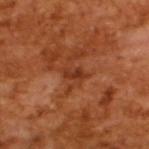Case summary:
• follow-up · catalogued during a skin exam; not biopsied
• subject · male, about 65 years old
• lesion size · ≈2.5 mm
• illumination · cross-polarized
• imaging modality · ~15 mm tile from a whole-body skin photo